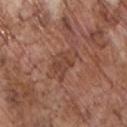The lesion was photographed on a routine skin check and not biopsied; there is no pathology result.
A 15 mm crop from a total-body photograph taken for skin-cancer surveillance.
Approximately 4 mm at its widest.
An algorithmic analysis of the crop reported an area of roughly 8 mm² and an eccentricity of roughly 0.8. And it measured internal color variation of about 3.5 on a 0–10 scale and a peripheral color-asymmetry measure near 1. The software also gave an automated nevus-likeness rating near 0 out of 100 and a detector confidence of about 100 out of 100 that the crop contains a lesion.
A male patient, aged around 70.
On the chest.
The tile uses white-light illumination.